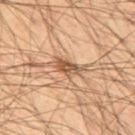Imaged during a routine full-body skin examination; the lesion was not biopsied and no histopathology is available.
The lesion is on the back.
Cropped from a whole-body photographic skin survey; the tile spans about 15 mm.
A male patient, in their mid-50s.
Captured under cross-polarized illumination.